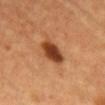Recorded during total-body skin imaging; not selected for excision or biopsy. Imaged with cross-polarized lighting. A female subject, aged approximately 40. About 4.5 mm across. The lesion-visualizer software estimated border irregularity of about 2 on a 0–10 scale, internal color variation of about 5.5 on a 0–10 scale, and peripheral color asymmetry of about 1.5. It also reported an automated nevus-likeness rating near 100 out of 100. A 15 mm close-up tile from a total-body photography series done for melanoma screening. The lesion is located on the chest.